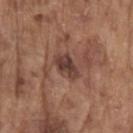Assessment:
The lesion was photographed on a routine skin check and not biopsied; there is no pathology result.
Background:
This image is a 15 mm lesion crop taken from a total-body photograph. Located on the left upper arm. A male subject roughly 80 years of age.